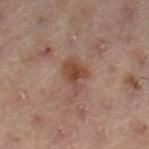Q: Was this lesion biopsied?
A: no biopsy performed (imaged during a skin exam)